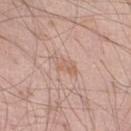Part of a total-body skin-imaging series; this lesion was reviewed on a skin check and was not flagged for biopsy. Automated image analysis of the tile measured a lesion area of about 5 mm², a shape eccentricity near 0.85, and a shape-asymmetry score of about 0.3 (0 = symmetric). And it measured a mean CIELAB color near L≈62 a*≈19 b*≈27 and a lesion–skin lightness drop of about 6. And it measured border irregularity of about 3.5 on a 0–10 scale and a within-lesion color-variation index near 3/10. And it measured a classifier nevus-likeness of about 0/100. About 3.5 mm across. A roughly 15 mm field-of-view crop from a total-body skin photograph. A male subject, aged 58 to 62. From the right lower leg. Captured under white-light illumination.